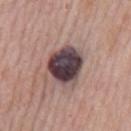Notes:
• notes — no biopsy performed (imaged during a skin exam)
• acquisition — ~15 mm crop, total-body skin-cancer survey
• site — the mid back
• lighting — white-light
• subject — male, in their mid-70s
• lesion size — ≈5 mm